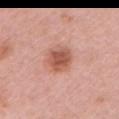Impression:
The lesion was photographed on a routine skin check and not biopsied; there is no pathology result.
Image and clinical context:
About 3.5 mm across. The tile uses white-light illumination. This image is a 15 mm lesion crop taken from a total-body photograph. A female subject, approximately 45 years of age. From the left upper arm. Automated tile analysis of the lesion measured a lesion color around L≈56 a*≈26 b*≈30 in CIELAB and a lesion-to-skin contrast of about 8.5 (normalized; higher = more distinct).